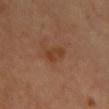| key | value |
|---|---|
| follow-up | catalogued during a skin exam; not biopsied |
| site | the front of the torso |
| patient | female, aged 38 to 42 |
| imaging modality | ~15 mm crop, total-body skin-cancer survey |
| automated lesion analysis | a footprint of about 4 mm² and two-axis asymmetry of about 0.3; about 7 CIELAB-L* units darker than the surrounding skin and a lesion-to-skin contrast of about 6.5 (normalized; higher = more distinct); a border-irregularity index near 2.5/10 and internal color variation of about 2 on a 0–10 scale |
| diameter | ≈2.5 mm |